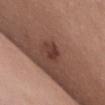This lesion was catalogued during total-body skin photography and was not selected for biopsy.
This image is a 15 mm lesion crop taken from a total-body photograph.
The lesion-visualizer software estimated a lesion area of about 4.5 mm², an outline eccentricity of about 0.75 (0 = round, 1 = elongated), and two-axis asymmetry of about 0.25. It also reported an average lesion color of about L≈38 a*≈21 b*≈24 (CIELAB), roughly 9 lightness units darker than nearby skin, and a lesion-to-skin contrast of about 7.5 (normalized; higher = more distinct). It also reported a peripheral color-asymmetry measure near 1.
The subject is a female aged around 65.
The lesion's longest dimension is about 3 mm.
The tile uses white-light illumination.
The lesion is located on the front of the torso.